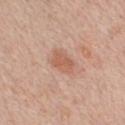No biopsy was performed on this lesion — it was imaged during a full skin examination and was not determined to be concerning. A roughly 15 mm field-of-view crop from a total-body skin photograph. The tile uses white-light illumination. A male patient, about 30 years old. Located on the chest. The recorded lesion diameter is about 3 mm.